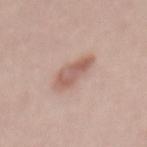This lesion was catalogued during total-body skin photography and was not selected for biopsy.
The lesion is on the back.
This is a white-light tile.
A 15 mm crop from a total-body photograph taken for skin-cancer surveillance.
Automated image analysis of the tile measured a footprint of about 7.5 mm² and an outline eccentricity of about 0.9 (0 = round, 1 = elongated). The analysis additionally found a border-irregularity rating of about 3/10, a color-variation rating of about 3.5/10, and a peripheral color-asymmetry measure near 1. The analysis additionally found an automated nevus-likeness rating near 45 out of 100 and a lesion-detection confidence of about 100/100.
A female subject aged 38 to 42.